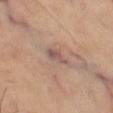Q: Is there a histopathology result?
A: imaged on a skin check; not biopsied
Q: What are the patient's age and sex?
A: male, roughly 65 years of age
Q: Automated lesion metrics?
A: a border-irregularity index near 4/10, internal color variation of about 2.5 on a 0–10 scale, and radial color variation of about 0.5; a classifier nevus-likeness of about 0/100 and a lesion-detection confidence of about 75/100
Q: Illumination type?
A: cross-polarized
Q: Lesion location?
A: the left thigh
Q: Lesion size?
A: about 3 mm
Q: What kind of image is this?
A: ~15 mm tile from a whole-body skin photo